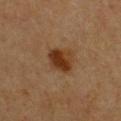Q: Is there a histopathology result?
A: imaged on a skin check; not biopsied
Q: Who is the patient?
A: female, aged 38 to 42
Q: Lesion size?
A: ≈3 mm
Q: Lesion location?
A: the front of the torso
Q: Illumination type?
A: cross-polarized illumination
Q: What is the imaging modality?
A: ~15 mm crop, total-body skin-cancer survey